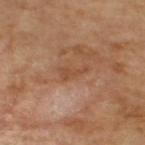Captured during whole-body skin photography for melanoma surveillance; the lesion was not biopsied.